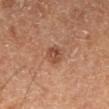Impression: No biopsy was performed on this lesion — it was imaged during a full skin examination and was not determined to be concerning. Context: Located on the left lower leg. A male patient, aged 58 to 62. A region of skin cropped from a whole-body photographic capture, roughly 15 mm wide.